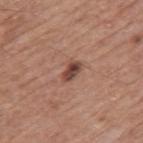Case summary:
* biopsy status · catalogued during a skin exam; not biopsied
* diameter · about 2.5 mm
* patient · male, in their mid-70s
* illumination · white-light illumination
* site · the mid back
* TBP lesion metrics · a lesion area of about 3.5 mm² and a shape-asymmetry score of about 0.2 (0 = symmetric)
* acquisition · 15 mm crop, total-body photography